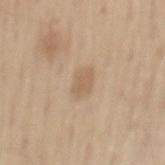notes: imaged on a skin check; not biopsied | site: the mid back | subject: male, aged 58–62 | image: ~15 mm tile from a whole-body skin photo | diameter: about 3.5 mm.